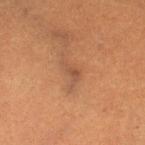Impression:
No biopsy was performed on this lesion — it was imaged during a full skin examination and was not determined to be concerning.
Acquisition and patient details:
The lesion is located on the left thigh. The lesion-visualizer software estimated an eccentricity of roughly 0.85 and two-axis asymmetry of about 0.45. It also reported a border-irregularity rating of about 4.5/10 and a peripheral color-asymmetry measure near 0. A 15 mm crop from a total-body photograph taken for skin-cancer surveillance. This is a cross-polarized tile. A female subject, roughly 55 years of age. Measured at roughly 2.5 mm in maximum diameter.